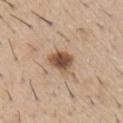The lesion was photographed on a routine skin check and not biopsied; there is no pathology result. The patient is a male aged approximately 60. From the right upper arm. Captured under white-light illumination. A close-up tile cropped from a whole-body skin photograph, about 15 mm across. Longest diameter approximately 3.5 mm.